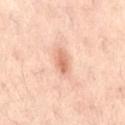notes: imaged on a skin check; not biopsied